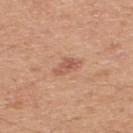The lesion was tiled from a total-body skin photograph and was not biopsied.
The lesion is on the upper back.
This is a white-light tile.
The lesion-visualizer software estimated an area of roughly 4 mm², an eccentricity of roughly 0.85, and a shape-asymmetry score of about 0.3 (0 = symmetric). And it measured a lesion color around L≈56 a*≈24 b*≈31 in CIELAB, about 9 CIELAB-L* units darker than the surrounding skin, and a normalized border contrast of about 6.5. It also reported a color-variation rating of about 3.5/10.
A lesion tile, about 15 mm wide, cut from a 3D total-body photograph.
Approximately 3 mm at its widest.
A male patient roughly 45 years of age.